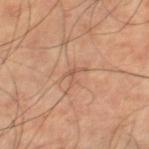* notes · no biopsy performed (imaged during a skin exam)
* image source · 15 mm crop, total-body photography
* size · about 2.5 mm
* site · the right thigh
* image-analysis metrics · a footprint of about 2.5 mm², an outline eccentricity of about 0.75 (0 = round, 1 = elongated), and two-axis asymmetry of about 0.7; an average lesion color of about L≈54 a*≈20 b*≈30 (CIELAB)
* patient · male, aged around 60